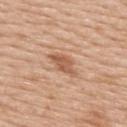Q: Is there a histopathology result?
A: catalogued during a skin exam; not biopsied
Q: How was this image acquired?
A: ~15 mm tile from a whole-body skin photo
Q: Lesion location?
A: the upper back
Q: Patient demographics?
A: female, in their mid-60s